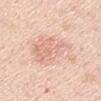Captured during whole-body skin photography for melanoma surveillance; the lesion was not biopsied.
A male patient, approximately 45 years of age.
About 6.5 mm across.
On the arm.
Automated tile analysis of the lesion measured a lesion color around L≈74 a*≈20 b*≈29 in CIELAB, roughly 8 lightness units darker than nearby skin, and a normalized lesion–skin contrast near 4.5. It also reported a border-irregularity index near 4/10, a within-lesion color-variation index near 4/10, and peripheral color asymmetry of about 1.5. The analysis additionally found a classifier nevus-likeness of about 0/100 and a detector confidence of about 100 out of 100 that the crop contains a lesion.
This is a white-light tile.
A 15 mm close-up extracted from a 3D total-body photography capture.